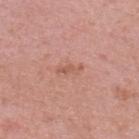<case>
  <biopsy_status>not biopsied; imaged during a skin examination</biopsy_status>
  <site>upper back</site>
  <automated_metrics>
    <nevus_likeness_0_100>0</nevus_likeness_0_100>
  </automated_metrics>
  <lighting>white-light</lighting>
  <patient>
    <sex>female</sex>
    <age_approx>40</age_approx>
  </patient>
  <image>
    <source>total-body photography crop</source>
    <field_of_view_mm>15</field_of_view_mm>
  </image>
  <lesion_size>
    <long_diameter_mm_approx>3.0</long_diameter_mm_approx>
  </lesion_size>
</case>